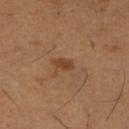This lesion was catalogued during total-body skin photography and was not selected for biopsy.
The recorded lesion diameter is about 2 mm.
This is a cross-polarized tile.
Located on the leg.
A roughly 15 mm field-of-view crop from a total-body skin photograph.
Automated image analysis of the tile measured an eccentricity of roughly 0.8. The software also gave a lesion color around L≈42 a*≈21 b*≈33 in CIELAB, a lesion–skin lightness drop of about 8, and a normalized border contrast of about 7. It also reported a classifier nevus-likeness of about 60/100.
A female patient aged approximately 55.